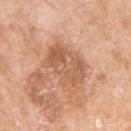Clinical impression:
No biopsy was performed on this lesion — it was imaged during a full skin examination and was not determined to be concerning.
Clinical summary:
A female subject, in their mid-70s. The total-body-photography lesion software estimated a lesion area of about 16 mm², a shape eccentricity near 0.75, and a symmetry-axis asymmetry near 0.2. The software also gave a lesion color around L≈59 a*≈23 b*≈34 in CIELAB and a lesion-to-skin contrast of about 6 (normalized; higher = more distinct). And it measured border irregularity of about 3 on a 0–10 scale, a color-variation rating of about 5/10, and radial color variation of about 1.5. Cropped from a total-body skin-imaging series; the visible field is about 15 mm. Captured under white-light illumination. From the left upper arm.